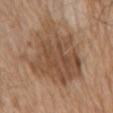Impression:
Imaged during a routine full-body skin examination; the lesion was not biopsied and no histopathology is available.
Context:
This is a white-light tile. A close-up tile cropped from a whole-body skin photograph, about 15 mm across. Longest diameter approximately 9.5 mm. Located on the chest. The subject is a male aged 68–72.